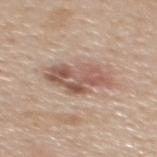Background:
A 15 mm crop from a total-body photograph taken for skin-cancer surveillance. From the mid back. The total-body-photography lesion software estimated a normalized border contrast of about 8. It also reported an automated nevus-likeness rating near 15 out of 100 and a lesion-detection confidence of about 100/100. A male patient, about 50 years old.